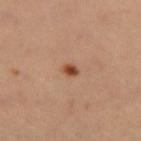Captured during whole-body skin photography for melanoma surveillance; the lesion was not biopsied. Located on the left thigh. A female subject, in their mid-30s. This image is a 15 mm lesion crop taken from a total-body photograph.